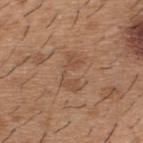On the upper back. This image is a 15 mm lesion crop taken from a total-body photograph. The total-body-photography lesion software estimated a lesion color around L≈50 a*≈19 b*≈31 in CIELAB and a lesion-to-skin contrast of about 5 (normalized; higher = more distinct). The patient is a male aged approximately 40. Approximately 4.5 mm at its widest.